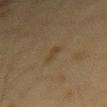Impression: Part of a total-body skin-imaging series; this lesion was reviewed on a skin check and was not flagged for biopsy. Clinical summary: Imaged with cross-polarized lighting. The subject is a female in their 40s. A lesion tile, about 15 mm wide, cut from a 3D total-body photograph. Measured at roughly 2.5 mm in maximum diameter. On the mid back.